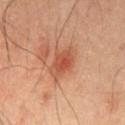No biopsy was performed on this lesion — it was imaged during a full skin examination and was not determined to be concerning. A close-up tile cropped from a whole-body skin photograph, about 15 mm across. The lesion-visualizer software estimated roughly 8 lightness units darker than nearby skin and a lesion-to-skin contrast of about 7 (normalized; higher = more distinct). It also reported lesion-presence confidence of about 100/100. The recorded lesion diameter is about 3.5 mm. The subject is a male approximately 50 years of age. Imaged with cross-polarized lighting. The lesion is located on the abdomen.